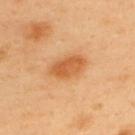biopsy status: total-body-photography surveillance lesion; no biopsy
body site: the upper back
patient: male, in their 40s
lesion size: ~4.5 mm (longest diameter)
image: ~15 mm crop, total-body skin-cancer survey
lighting: cross-polarized illumination